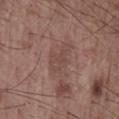This lesion was catalogued during total-body skin photography and was not selected for biopsy.
Captured under white-light illumination.
A close-up tile cropped from a whole-body skin photograph, about 15 mm across.
Located on the chest.
Measured at roughly 4 mm in maximum diameter.
A male patient approximately 60 years of age.